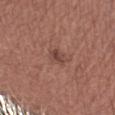Findings:
• notes · total-body-photography surveillance lesion; no biopsy
• patient · male, in their mid-50s
• imaging modality · total-body-photography crop, ~15 mm field of view
• location · the right forearm